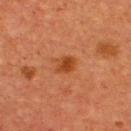| key | value |
|---|---|
| follow-up | no biopsy performed (imaged during a skin exam) |
| lesion diameter | ~2.5 mm (longest diameter) |
| automated lesion analysis | a lesion area of about 4.5 mm², a shape eccentricity near 0.6, and two-axis asymmetry of about 0.2; an average lesion color of about L≈42 a*≈28 b*≈39 (CIELAB), a lesion–skin lightness drop of about 9, and a lesion-to-skin contrast of about 8 (normalized; higher = more distinct); a nevus-likeness score of about 95/100 |
| patient | male, in their mid-60s |
| imaging modality | ~15 mm tile from a whole-body skin photo |
| lighting | cross-polarized illumination |
| site | the chest |